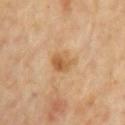follow-up = no biopsy performed (imaged during a skin exam) | site = the right upper arm | diameter = about 3 mm | subject = male, about 65 years old | illumination = cross-polarized | acquisition = ~15 mm crop, total-body skin-cancer survey.